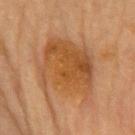  biopsy_status: not biopsied; imaged during a skin examination
  lesion_size:
    long_diameter_mm_approx: 7.0
  patient:
    sex: female
    age_approx: 60
  site: front of the torso
  lighting: cross-polarized
  automated_metrics:
    cielab_L: 45
    cielab_a: 20
    cielab_b: 36
    vs_skin_darker_L: 8.0
    vs_skin_contrast_norm: 7.0
    border_irregularity_0_10: 4.0
    color_variation_0_10: 5.0
    peripheral_color_asymmetry: 2.0
  image:
    source: total-body photography crop
    field_of_view_mm: 15